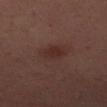Recorded during total-body skin imaging; not selected for excision or biopsy. A female subject approximately 40 years of age. The lesion is located on the left upper arm. About 3 mm across. Imaged with cross-polarized lighting. A region of skin cropped from a whole-body photographic capture, roughly 15 mm wide. The total-body-photography lesion software estimated an area of roughly 5.5 mm², a shape eccentricity near 0.7, and two-axis asymmetry of about 0.25. And it measured a mean CIELAB color near L≈28 a*≈20 b*≈21 and a normalized border contrast of about 6.5. The analysis additionally found a color-variation rating of about 2/10. And it measured a nevus-likeness score of about 55/100.